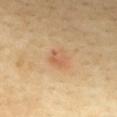notes: no biopsy performed (imaged during a skin exam)
automated lesion analysis: a footprint of about 4.5 mm², an outline eccentricity of about 0.6 (0 = round, 1 = elongated), and a shape-asymmetry score of about 0.3 (0 = symmetric); a classifier nevus-likeness of about 0/100 and a lesion-detection confidence of about 100/100
size: ~2.5 mm (longest diameter)
tile lighting: cross-polarized
image source: total-body-photography crop, ~15 mm field of view
location: the front of the torso
patient: female, roughly 55 years of age